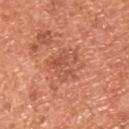Impression:
The lesion was photographed on a routine skin check and not biopsied; there is no pathology result.
Background:
The tile uses white-light illumination. Longest diameter approximately 4 mm. The lesion-visualizer software estimated a color-variation rating of about 3.5/10. And it measured a detector confidence of about 100 out of 100 that the crop contains a lesion. This image is a 15 mm lesion crop taken from a total-body photograph. The lesion is on the back. A male subject about 55 years old.